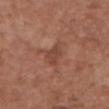Q: Was this lesion biopsied?
A: total-body-photography surveillance lesion; no biopsy
Q: Lesion location?
A: the chest
Q: What is the imaging modality?
A: ~15 mm tile from a whole-body skin photo
Q: What are the patient's age and sex?
A: female, in their mid-70s